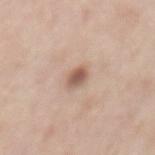Captured during whole-body skin photography for melanoma surveillance; the lesion was not biopsied. The lesion is located on the chest. A male patient roughly 60 years of age. Imaged with white-light lighting. An algorithmic analysis of the crop reported a shape eccentricity near 0.65. The analysis additionally found a color-variation rating of about 3/10 and peripheral color asymmetry of about 1. It also reported a nevus-likeness score of about 80/100 and a detector confidence of about 100 out of 100 that the crop contains a lesion. About 2.5 mm across. A 15 mm crop from a total-body photograph taken for skin-cancer surveillance.